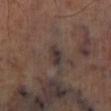Q: Is there a histopathology result?
A: no biopsy performed (imaged during a skin exam)
Q: Illumination type?
A: cross-polarized
Q: Automated lesion metrics?
A: an outline eccentricity of about 0.85 (0 = round, 1 = elongated) and two-axis asymmetry of about 0.2; border irregularity of about 2 on a 0–10 scale, internal color variation of about 2.5 on a 0–10 scale, and a peripheral color-asymmetry measure near 1; a detector confidence of about 60 out of 100 that the crop contains a lesion
Q: What is the lesion's diameter?
A: about 2.5 mm
Q: Lesion location?
A: the right lower leg
Q: How was this image acquired?
A: total-body-photography crop, ~15 mm field of view